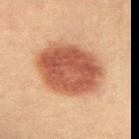Part of a total-body skin-imaging series; this lesion was reviewed on a skin check and was not flagged for biopsy. Longest diameter approximately 7.5 mm. A lesion tile, about 15 mm wide, cut from a 3D total-body photograph. A female subject aged 53 to 57. From the left forearm.